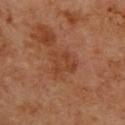This lesion was catalogued during total-body skin photography and was not selected for biopsy.
Measured at roughly 3.5 mm in maximum diameter.
The lesion is on the upper back.
A male subject, aged 58 to 62.
This is a cross-polarized tile.
Cropped from a whole-body photographic skin survey; the tile spans about 15 mm.